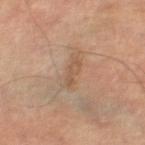{
  "biopsy_status": "not biopsied; imaged during a skin examination",
  "image": {
    "source": "total-body photography crop",
    "field_of_view_mm": 15
  },
  "patient": {
    "sex": "male",
    "age_approx": 65
  },
  "site": "right lower leg",
  "lighting": "cross-polarized",
  "automated_metrics": {
    "area_mm2_approx": 3.5,
    "eccentricity": 0.95,
    "shape_asymmetry": 0.35,
    "cielab_L": 51,
    "cielab_a": 17,
    "cielab_b": 30,
    "vs_skin_contrast_norm": 5.5,
    "nevus_likeness_0_100": 0,
    "lesion_detection_confidence_0_100": 100
  },
  "lesion_size": {
    "long_diameter_mm_approx": 3.5
  }
}